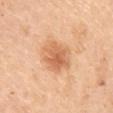Impression:
Imaged during a routine full-body skin examination; the lesion was not biopsied and no histopathology is available.
Acquisition and patient details:
The lesion is on the arm. A female patient, in their mid-60s. A region of skin cropped from a whole-body photographic capture, roughly 15 mm wide.